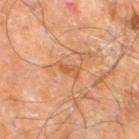Clinical impression: This lesion was catalogued during total-body skin photography and was not selected for biopsy. Image and clinical context: A roughly 15 mm field-of-view crop from a total-body skin photograph. A male patient about 60 years old. From the right leg. Imaged with cross-polarized lighting. The lesion's longest dimension is about 3 mm. The lesion-visualizer software estimated a mean CIELAB color near L≈56 a*≈25 b*≈40.